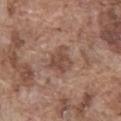No biopsy was performed on this lesion — it was imaged during a full skin examination and was not determined to be concerning.
The lesion is on the abdomen.
A lesion tile, about 15 mm wide, cut from a 3D total-body photograph.
Measured at roughly 3.5 mm in maximum diameter.
This is a white-light tile.
A male subject roughly 75 years of age.